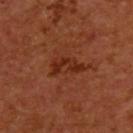{
  "biopsy_status": "not biopsied; imaged during a skin examination",
  "image": {
    "source": "total-body photography crop",
    "field_of_view_mm": 15
  },
  "site": "upper back",
  "patient": {
    "sex": "male",
    "age_approx": 55
  }
}